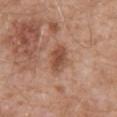Imaged during a routine full-body skin examination; the lesion was not biopsied and no histopathology is available. Cropped from a whole-body photographic skin survey; the tile spans about 15 mm. Automated image analysis of the tile measured an eccentricity of roughly 0.8 and two-axis asymmetry of about 0.25. And it measured a border-irregularity rating of about 2.5/10, a color-variation rating of about 4.5/10, and radial color variation of about 1.5. And it measured a nevus-likeness score of about 50/100 and lesion-presence confidence of about 100/100. Measured at roughly 4 mm in maximum diameter. This is a white-light tile. The subject is a male aged approximately 60. The lesion is located on the mid back.